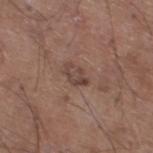{
  "biopsy_status": "not biopsied; imaged during a skin examination",
  "lesion_size": {
    "long_diameter_mm_approx": 3.0
  },
  "image": {
    "source": "total-body photography crop",
    "field_of_view_mm": 15
  },
  "patient": {
    "sex": "male",
    "age_approx": 60
  },
  "lighting": "white-light",
  "site": "left lower leg",
  "automated_metrics": {
    "area_mm2_approx": 3.0,
    "eccentricity": 0.8,
    "cielab_L": 42,
    "cielab_a": 18,
    "cielab_b": 22,
    "vs_skin_darker_L": 9.0,
    "vs_skin_contrast_norm": 7.0,
    "border_irregularity_0_10": 8.5,
    "color_variation_0_10": 0.0,
    "peripheral_color_asymmetry": 0.0,
    "nevus_likeness_0_100": 0,
    "lesion_detection_confidence_0_100": 100
  }
}